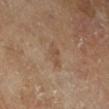Imaged during a routine full-body skin examination; the lesion was not biopsied and no histopathology is available.
A close-up tile cropped from a whole-body skin photograph, about 15 mm across.
The recorded lesion diameter is about 3 mm.
Located on the right lower leg.
The tile uses cross-polarized illumination.
A male patient, about 65 years old.